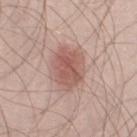{
  "biopsy_status": "not biopsied; imaged during a skin examination",
  "site": "left thigh",
  "lesion_size": {
    "long_diameter_mm_approx": 5.0
  },
  "lighting": "white-light",
  "patient": {
    "sex": "male",
    "age_approx": 35
  },
  "automated_metrics": {
    "vs_skin_contrast_norm": 7.0,
    "color_variation_0_10": 3.5,
    "peripheral_color_asymmetry": 1.0
  },
  "image": {
    "source": "total-body photography crop",
    "field_of_view_mm": 15
  }
}